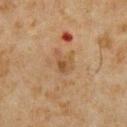biopsy status = imaged on a skin check; not biopsied | subject = male, about 60 years old | lesion diameter = ≈3 mm | illumination = cross-polarized | imaging modality = 15 mm crop, total-body photography | automated lesion analysis = an area of roughly 4 mm², an eccentricity of roughly 0.7, and two-axis asymmetry of about 0.4; a border-irregularity rating of about 4.5/10 and internal color variation of about 3 on a 0–10 scale; a classifier nevus-likeness of about 0/100 and lesion-presence confidence of about 100/100 | anatomic site = the chest.